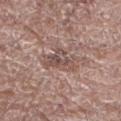{
  "biopsy_status": "not biopsied; imaged during a skin examination",
  "automated_metrics": {
    "area_mm2_approx": 8.0,
    "eccentricity": 0.7,
    "shape_asymmetry": 0.4,
    "vs_skin_darker_L": 9.0,
    "vs_skin_contrast_norm": 6.5
  },
  "site": "leg",
  "image": {
    "source": "total-body photography crop",
    "field_of_view_mm": 15
  },
  "lighting": "white-light",
  "patient": {
    "sex": "male",
    "age_approx": 70
  },
  "lesion_size": {
    "long_diameter_mm_approx": 4.0
  }
}